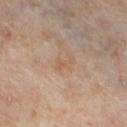Part of a total-body skin-imaging series; this lesion was reviewed on a skin check and was not flagged for biopsy.
The lesion is on the right lower leg.
The patient is a female about 50 years old.
This is a cross-polarized tile.
Approximately 2.5 mm at its widest.
A roughly 15 mm field-of-view crop from a total-body skin photograph.
Automated tile analysis of the lesion measured a footprint of about 2 mm², an outline eccentricity of about 0.9 (0 = round, 1 = elongated), and a shape-asymmetry score of about 0.6 (0 = symmetric). The software also gave a border-irregularity rating of about 6.5/10 and a within-lesion color-variation index near 0/10.